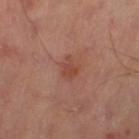The lesion was tiled from a total-body skin photograph and was not biopsied. Automated tile analysis of the lesion measured a mean CIELAB color near L≈46 a*≈26 b*≈28, about 7 CIELAB-L* units darker than the surrounding skin, and a normalized lesion–skin contrast near 6. The lesion is on the leg. A close-up tile cropped from a whole-body skin photograph, about 15 mm across. Imaged with cross-polarized lighting.